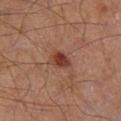Q: Was a biopsy performed?
A: total-body-photography surveillance lesion; no biopsy
Q: What is the anatomic site?
A: the right lower leg
Q: Who is the patient?
A: male, about 65 years old
Q: What did automated image analysis measure?
A: a footprint of about 4.5 mm², a shape eccentricity near 0.75, and a symmetry-axis asymmetry near 0.15; a border-irregularity rating of about 1.5/10, a within-lesion color-variation index near 2.5/10, and a peripheral color-asymmetry measure near 1
Q: What kind of image is this?
A: total-body-photography crop, ~15 mm field of view
Q: How was the tile lit?
A: cross-polarized
Q: Lesion size?
A: ~3 mm (longest diameter)